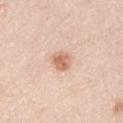Case summary:
- workup: no biopsy performed (imaged during a skin exam)
- patient: male, aged 43 to 47
- illumination: white-light illumination
- imaging modality: ~15 mm tile from a whole-body skin photo
- anatomic site: the right upper arm